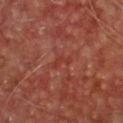<record>
<biopsy_status>not biopsied; imaged during a skin examination</biopsy_status>
<site>front of the torso</site>
<image>
  <source>total-body photography crop</source>
  <field_of_view_mm>15</field_of_view_mm>
</image>
<automated_metrics>
  <area_mm2_approx>2.0</area_mm2_approx>
  <eccentricity>0.9</eccentricity>
  <shape_asymmetry>0.6</shape_asymmetry>
  <cielab_L>33</cielab_L>
  <cielab_a>28</cielab_a>
  <cielab_b>27</cielab_b>
  <vs_skin_darker_L>4.0</vs_skin_darker_L>
  <vs_skin_contrast_norm>4.5</vs_skin_contrast_norm>
  <nevus_likeness_0_100>0</nevus_likeness_0_100>
</automated_metrics>
<patient>
  <sex>male</sex>
  <age_approx>65</age_approx>
</patient>
<lighting>cross-polarized</lighting>
</record>